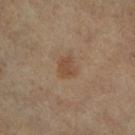Q: What kind of image is this?
A: ~15 mm crop, total-body skin-cancer survey
Q: Where on the body is the lesion?
A: the left leg
Q: Patient demographics?
A: female, about 65 years old